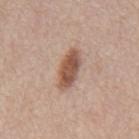Imaged during a routine full-body skin examination; the lesion was not biopsied and no histopathology is available. On the mid back. A roughly 15 mm field-of-view crop from a total-body skin photograph. A male subject in their mid-60s. The total-body-photography lesion software estimated a shape-asymmetry score of about 0.25 (0 = symmetric). The analysis additionally found a lesion color around L≈53 a*≈20 b*≈28 in CIELAB, a lesion–skin lightness drop of about 14, and a normalized lesion–skin contrast near 10. It also reported border irregularity of about 2.5 on a 0–10 scale. The lesion's longest dimension is about 4.5 mm. This is a white-light tile.